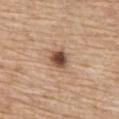Imaged during a routine full-body skin examination; the lesion was not biopsied and no histopathology is available. The lesion is located on the chest. The subject is a male in their 70s. Measured at roughly 2.5 mm in maximum diameter. Automated image analysis of the tile measured a shape-asymmetry score of about 0.15 (0 = symmetric). The software also gave a mean CIELAB color near L≈49 a*≈20 b*≈29 and a lesion–skin lightness drop of about 17. Imaged with white-light lighting. A lesion tile, about 15 mm wide, cut from a 3D total-body photograph.